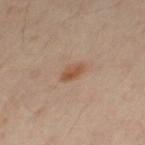patient=female, about 40 years old | automated metrics=a lesion area of about 3.5 mm², an eccentricity of roughly 0.85, and a symmetry-axis asymmetry near 0.25; internal color variation of about 2.5 on a 0–10 scale; a classifier nevus-likeness of about 75/100 | tile lighting=cross-polarized illumination | body site=the right leg | image source=total-body-photography crop, ~15 mm field of view.